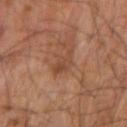| field | value |
|---|---|
| notes | total-body-photography surveillance lesion; no biopsy |
| subject | male, about 60 years old |
| illumination | cross-polarized illumination |
| site | the arm |
| imaging modality | ~15 mm tile from a whole-body skin photo |